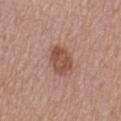Impression: No biopsy was performed on this lesion — it was imaged during a full skin examination and was not determined to be concerning. Clinical summary: The lesion is on the left thigh. This is a white-light tile. A region of skin cropped from a whole-body photographic capture, roughly 15 mm wide. The subject is a female aged around 55.